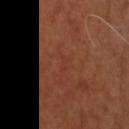Clinical impression:
The lesion was photographed on a routine skin check and not biopsied; there is no pathology result.
Image and clinical context:
This is a cross-polarized tile. On the head or neck. A roughly 15 mm field-of-view crop from a total-body skin photograph. A male subject approximately 55 years of age. Longest diameter approximately 1.5 mm.